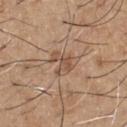Image and clinical context:
A male patient roughly 65 years of age. This is a white-light tile. The recorded lesion diameter is about 3.5 mm. A close-up tile cropped from a whole-body skin photograph, about 15 mm across. From the chest. Automated tile analysis of the lesion measured a footprint of about 7 mm² and a shape eccentricity near 0.45. It also reported an average lesion color of about L≈52 a*≈18 b*≈29 (CIELAB). It also reported an automated nevus-likeness rating near 0 out of 100 and a detector confidence of about 100 out of 100 that the crop contains a lesion.